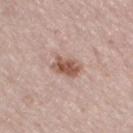This lesion was catalogued during total-body skin photography and was not selected for biopsy. The tile uses white-light illumination. Cropped from a whole-body photographic skin survey; the tile spans about 15 mm. From the right thigh. The patient is a male aged approximately 65. About 3.5 mm across.